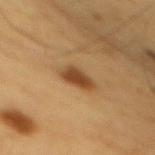notes = no biopsy performed (imaged during a skin exam) | acquisition = ~15 mm crop, total-body skin-cancer survey | automated lesion analysis = a border-irregularity rating of about 3/10 and a peripheral color-asymmetry measure near 0.5; a classifier nevus-likeness of about 100/100 and a detector confidence of about 100 out of 100 that the crop contains a lesion | anatomic site = the mid back | lighting = cross-polarized illumination | lesion diameter = ≈4 mm | patient = male, approximately 60 years of age.